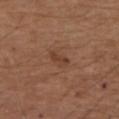follow-up: imaged on a skin check; not biopsied | subject: male, aged 73 to 77 | image source: total-body-photography crop, ~15 mm field of view | site: the upper back | lighting: white-light illumination.